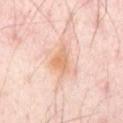  biopsy_status: not biopsied; imaged during a skin examination
  image:
    source: total-body photography crop
    field_of_view_mm: 15
  patient:
    sex: male
    age_approx: 50
  site: abdomen